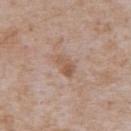Assessment:
This lesion was catalogued during total-body skin photography and was not selected for biopsy.
Background:
A lesion tile, about 15 mm wide, cut from a 3D total-body photograph. The total-body-photography lesion software estimated a border-irregularity index near 3.5/10 and a color-variation rating of about 4/10. The software also gave lesion-presence confidence of about 100/100. A male patient, roughly 65 years of age. The lesion is on the chest. This is a white-light tile.